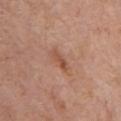Clinical impression: The lesion was tiled from a total-body skin photograph and was not biopsied. Background: Imaged with white-light lighting. The lesion is located on the chest. The recorded lesion diameter is about 3 mm. The subject is a male aged 78–82. The lesion-visualizer software estimated a detector confidence of about 100 out of 100 that the crop contains a lesion. A close-up tile cropped from a whole-body skin photograph, about 15 mm across.